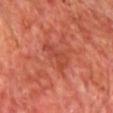The lesion was photographed on a routine skin check and not biopsied; there is no pathology result. The total-body-photography lesion software estimated a footprint of about 5 mm² and a shape-asymmetry score of about 0.45 (0 = symmetric). The analysis additionally found a border-irregularity index near 5.5/10, internal color variation of about 1.5 on a 0–10 scale, and a peripheral color-asymmetry measure near 0.5. And it measured a nevus-likeness score of about 0/100 and lesion-presence confidence of about 100/100. A roughly 15 mm field-of-view crop from a total-body skin photograph. The tile uses cross-polarized illumination. The patient is a male about 60 years old. Measured at roughly 3.5 mm in maximum diameter. The lesion is on the chest.